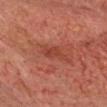  biopsy_status: not biopsied; imaged during a skin examination
  automated_metrics:
    area_mm2_approx: 5.0
    eccentricity: 0.85
    shape_asymmetry: 0.4
    cielab_L: 35
    cielab_a: 25
    cielab_b: 26
    vs_skin_darker_L: 6.0
    vs_skin_contrast_norm: 5.5
    nevus_likeness_0_100: 0
    lesion_detection_confidence_0_100: 90
  image:
    source: total-body photography crop
    field_of_view_mm: 15
  patient:
    sex: male
    age_approx: 75
  site: head or neck
  lighting: cross-polarized